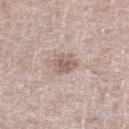Assessment: Imaged during a routine full-body skin examination; the lesion was not biopsied and no histopathology is available. Clinical summary: The lesion-visualizer software estimated a border-irregularity rating of about 2.5/10, a within-lesion color-variation index near 2/10, and peripheral color asymmetry of about 0.5. On the leg. Cropped from a total-body skin-imaging series; the visible field is about 15 mm. A male subject, in their mid- to late 60s. Captured under white-light illumination.